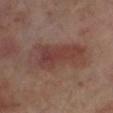Q: Was this lesion biopsied?
A: total-body-photography surveillance lesion; no biopsy
Q: What is the anatomic site?
A: the leg
Q: What are the patient's age and sex?
A: male, aged 68–72
Q: How large is the lesion?
A: about 8 mm
Q: Illumination type?
A: cross-polarized illumination
Q: What is the imaging modality?
A: ~15 mm crop, total-body skin-cancer survey